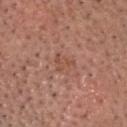biopsy status: total-body-photography surveillance lesion; no biopsy | anatomic site: the head or neck | patient: male, aged 58–62 | image source: ~15 mm crop, total-body skin-cancer survey.